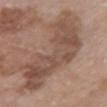<case>
  <biopsy_status>not biopsied; imaged during a skin examination</biopsy_status>
  <site>chest</site>
  <lighting>white-light</lighting>
  <automated_metrics>
    <area_mm2_approx>39.0</area_mm2_approx>
    <eccentricity>0.95</eccentricity>
    <shape_asymmetry>0.45</shape_asymmetry>
    <cielab_L>49</cielab_L>
    <cielab_a>18</cielab_a>
    <cielab_b>26</cielab_b>
    <vs_skin_darker_L>10.0</vs_skin_darker_L>
    <vs_skin_contrast_norm>7.5</vs_skin_contrast_norm>
    <nevus_likeness_0_100>0</nevus_likeness_0_100>
  </automated_metrics>
  <patient>
    <sex>female</sex>
    <age_approx>70</age_approx>
  </patient>
  <lesion_size>
    <long_diameter_mm_approx>12.0</long_diameter_mm_approx>
  </lesion_size>
  <image>
    <source>total-body photography crop</source>
    <field_of_view_mm>15</field_of_view_mm>
  </image>
</case>